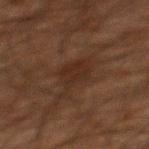<case>
<biopsy_status>not biopsied; imaged during a skin examination</biopsy_status>
<lesion_size>
  <long_diameter_mm_approx>5.5</long_diameter_mm_approx>
</lesion_size>
<patient>
  <sex>male</sex>
  <age_approx>60</age_approx>
</patient>
<automated_metrics>
  <area_mm2_approx>9.0</area_mm2_approx>
  <eccentricity>0.85</eccentricity>
  <shape_asymmetry>0.45</shape_asymmetry>
  <border_irregularity_0_10>5.5</border_irregularity_0_10>
  <peripheral_color_asymmetry>0.5</peripheral_color_asymmetry>
</automated_metrics>
<image>
  <source>total-body photography crop</source>
  <field_of_view_mm>15</field_of_view_mm>
</image>
<lighting>cross-polarized</lighting>
<site>mid back</site>
</case>